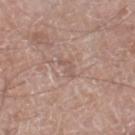| key | value |
|---|---|
| biopsy status | total-body-photography surveillance lesion; no biopsy |
| tile lighting | white-light illumination |
| automated metrics | an average lesion color of about L≈55 a*≈18 b*≈24 (CIELAB) and a lesion-to-skin contrast of about 5 (normalized; higher = more distinct); a border-irregularity rating of about 6/10, a color-variation rating of about 0/10, and a peripheral color-asymmetry measure near 0 |
| site | the leg |
| size | about 3 mm |
| subject | male, approximately 65 years of age |
| image source | 15 mm crop, total-body photography |